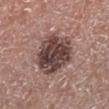Clinical impression:
Captured during whole-body skin photography for melanoma surveillance; the lesion was not biopsied.
Acquisition and patient details:
On the right lower leg. The patient is a male aged 53 to 57. A close-up tile cropped from a whole-body skin photograph, about 15 mm across. The lesion's longest dimension is about 6 mm. Captured under white-light illumination.